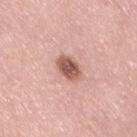biopsy status: no biopsy performed (imaged during a skin exam)
acquisition: ~15 mm crop, total-body skin-cancer survey
location: the right thigh
subject: female, roughly 50 years of age